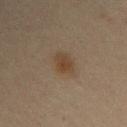imaging modality: total-body-photography crop, ~15 mm field of view
lesion size: ≈3 mm
subject: male, in their mid-30s
body site: the right upper arm